A male patient roughly 65 years of age · a 15 mm close-up extracted from a 3D total-body photography capture · the lesion is on the head or neck: 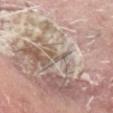Histopathological examination showed an invasive squamous cell carcinoma (malignant).